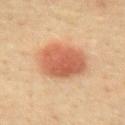The lesion was photographed on a routine skin check and not biopsied; there is no pathology result. Imaged with cross-polarized lighting. The patient is a male in their 40s. Approximately 6 mm at its widest. A 15 mm close-up tile from a total-body photography series done for melanoma screening. On the mid back.